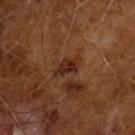image:
  source: total-body photography crop
  field_of_view_mm: 15
lighting: cross-polarized
lesion_size:
  long_diameter_mm_approx: 3.5
patient:
  sex: male
  age_approx: 65